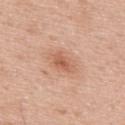workup = catalogued during a skin exam; not biopsied | lesion size = ≈3 mm | subject = male, roughly 65 years of age | anatomic site = the upper back | imaging modality = total-body-photography crop, ~15 mm field of view.